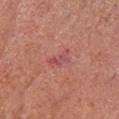{"biopsy_status": "not biopsied; imaged during a skin examination", "patient": {"sex": "male", "age_approx": 65}, "lighting": "white-light", "image": {"source": "total-body photography crop", "field_of_view_mm": 15}, "lesion_size": {"long_diameter_mm_approx": 3.5}, "automated_metrics": {"area_mm2_approx": 4.0, "eccentricity": 0.9, "shape_asymmetry": 0.45, "color_variation_0_10": 5.0, "peripheral_color_asymmetry": 1.5}, "site": "head or neck"}